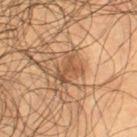Findings:
– follow-up · imaged on a skin check; not biopsied
– size · ≈4 mm
– location · the right lower leg
– image source · ~15 mm crop, total-body skin-cancer survey
– automated metrics · a footprint of about 7 mm², a shape eccentricity near 0.8, and a shape-asymmetry score of about 0.5 (0 = symmetric); a lesion color around L≈48 a*≈19 b*≈32 in CIELAB, about 9 CIELAB-L* units darker than the surrounding skin, and a normalized border contrast of about 7
– patient · male, aged 53–57